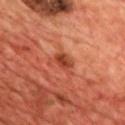notes: no biopsy performed (imaged during a skin exam) | lesion diameter: ≈3 mm | patient: male, roughly 70 years of age | imaging modality: total-body-photography crop, ~15 mm field of view | location: the chest | lighting: cross-polarized.